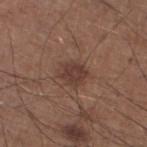Assessment:
Recorded during total-body skin imaging; not selected for excision or biopsy.
Clinical summary:
The recorded lesion diameter is about 3 mm. Captured under white-light illumination. A 15 mm close-up tile from a total-body photography series done for melanoma screening. Located on the left lower leg. Automated image analysis of the tile measured an area of roughly 6.5 mm², an eccentricity of roughly 0.55, and a symmetry-axis asymmetry near 0.2. The analysis additionally found border irregularity of about 2 on a 0–10 scale, internal color variation of about 2 on a 0–10 scale, and peripheral color asymmetry of about 0.5. A male patient approximately 60 years of age.